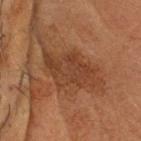A male subject, roughly 65 years of age. The lesion is located on the head or neck. The total-body-photography lesion software estimated a lesion area of about 17 mm² and an eccentricity of roughly 0.85. It also reported a mean CIELAB color near L≈32 a*≈19 b*≈27, roughly 6 lightness units darker than nearby skin, and a normalized lesion–skin contrast near 6. The software also gave a nevus-likeness score of about 0/100 and lesion-presence confidence of about 95/100. Cropped from a total-body skin-imaging series; the visible field is about 15 mm. The recorded lesion diameter is about 6.5 mm.